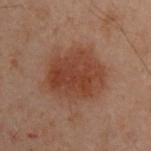Assessment: Imaged during a routine full-body skin examination; the lesion was not biopsied and no histopathology is available. Context: Imaged with cross-polarized lighting. A male patient aged 48–52. On the left upper arm. Longest diameter approximately 6.5 mm. A 15 mm crop from a total-body photograph taken for skin-cancer surveillance.